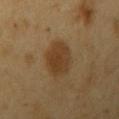workup: total-body-photography surveillance lesion; no biopsy
subject: male, aged 58 to 62
acquisition: ~15 mm tile from a whole-body skin photo
body site: the right upper arm
diameter: ~4.5 mm (longest diameter)
tile lighting: cross-polarized